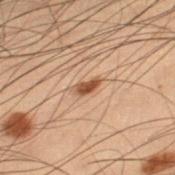Part of a total-body skin-imaging series; this lesion was reviewed on a skin check and was not flagged for biopsy. The lesion is located on the right thigh. This is a cross-polarized tile. A lesion tile, about 15 mm wide, cut from a 3D total-body photograph. The recorded lesion diameter is about 2.5 mm. The patient is a male in their mid-50s.